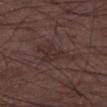| key | value |
|---|---|
| biopsy status | total-body-photography surveillance lesion; no biopsy |
| diameter | ≈5 mm |
| image | 15 mm crop, total-body photography |
| illumination | white-light |
| location | the left thigh |
| subject | male, about 50 years old |